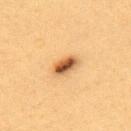This lesion was catalogued during total-body skin photography and was not selected for biopsy.